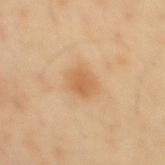{"biopsy_status": "not biopsied; imaged during a skin examination", "patient": {"sex": "male", "age_approx": 40}, "image": {"source": "total-body photography crop", "field_of_view_mm": 15}, "lighting": "cross-polarized", "automated_metrics": {"area_mm2_approx": 7.5, "eccentricity": 0.55, "vs_skin_darker_L": 8.0, "vs_skin_contrast_norm": 6.0, "lesion_detection_confidence_0_100": 100}, "site": "mid back", "lesion_size": {"long_diameter_mm_approx": 3.0}}